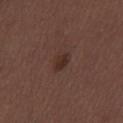{
  "biopsy_status": "not biopsied; imaged during a skin examination",
  "patient": {
    "sex": "male",
    "age_approx": 30
  },
  "site": "back",
  "image": {
    "source": "total-body photography crop",
    "field_of_view_mm": 15
  },
  "lighting": "white-light",
  "lesion_size": {
    "long_diameter_mm_approx": 2.5
  }
}